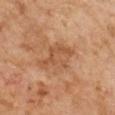<case>
<image>
  <source>total-body photography crop</source>
  <field_of_view_mm>15</field_of_view_mm>
</image>
<lighting>cross-polarized</lighting>
<patient>
  <sex>male</sex>
  <age_approx>65</age_approx>
</patient>
<lesion_size>
  <long_diameter_mm_approx>5.0</long_diameter_mm_approx>
</lesion_size>
</case>